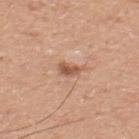Impression: Part of a total-body skin-imaging series; this lesion was reviewed on a skin check and was not flagged for biopsy. Acquisition and patient details: The tile uses white-light illumination. Cropped from a total-body skin-imaging series; the visible field is about 15 mm. The lesion is on the upper back. The recorded lesion diameter is about 3 mm. A male patient approximately 45 years of age.